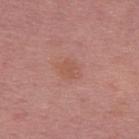This lesion was catalogued during total-body skin photography and was not selected for biopsy. Automated image analysis of the tile measured a lesion area of about 5 mm², a shape eccentricity near 0.7, and a shape-asymmetry score of about 0.2 (0 = symmetric). It also reported a classifier nevus-likeness of about 5/100 and a lesion-detection confidence of about 100/100. Longest diameter approximately 3 mm. The lesion is on the upper back. Imaged with white-light lighting. A male patient, aged 53–57. A 15 mm close-up tile from a total-body photography series done for melanoma screening.